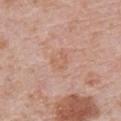biopsy status: total-body-photography surveillance lesion; no biopsy
image source: 15 mm crop, total-body photography
body site: the chest
lighting: white-light illumination
patient: male, aged around 70
TBP lesion metrics: a lesion area of about 4.5 mm² and an eccentricity of roughly 0.55; border irregularity of about 2 on a 0–10 scale, a color-variation rating of about 1.5/10, and peripheral color asymmetry of about 0.5